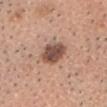Q: Was a biopsy performed?
A: total-body-photography surveillance lesion; no biopsy
Q: How large is the lesion?
A: about 4 mm
Q: What did automated image analysis measure?
A: border irregularity of about 2.5 on a 0–10 scale and a within-lesion color-variation index near 4.5/10
Q: What is the anatomic site?
A: the head or neck
Q: Illumination type?
A: white-light illumination
Q: Who is the patient?
A: male, approximately 60 years of age
Q: What kind of image is this?
A: 15 mm crop, total-body photography